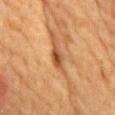No biopsy was performed on this lesion — it was imaged during a full skin examination and was not determined to be concerning. A lesion tile, about 15 mm wide, cut from a 3D total-body photograph. On the chest. Approximately 3 mm at its widest. A male patient in their mid-80s or older.